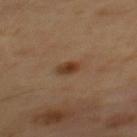Q: How was this image acquired?
A: total-body-photography crop, ~15 mm field of view
Q: Who is the patient?
A: male, about 65 years old
Q: Where on the body is the lesion?
A: the mid back